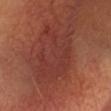| key | value |
|---|---|
| patient | male, in their mid-50s |
| acquisition | 15 mm crop, total-body photography |
| lighting | cross-polarized illumination |
| automated lesion analysis | a lesion area of about 23 mm², an eccentricity of roughly 0.6, and a shape-asymmetry score of about 0.5 (0 = symmetric); a lesion color around L≈35 a*≈26 b*≈25 in CIELAB, roughly 5 lightness units darker than nearby skin, and a normalized border contrast of about 5; border irregularity of about 8.5 on a 0–10 scale, internal color variation of about 2.5 on a 0–10 scale, and a peripheral color-asymmetry measure near 1; a nevus-likeness score of about 5/100 |
| location | the head or neck |